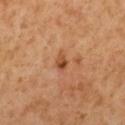Clinical impression:
The lesion was tiled from a total-body skin photograph and was not biopsied.
Clinical summary:
A male patient, in their 60s. The total-body-photography lesion software estimated a shape eccentricity near 0.8. The analysis additionally found a lesion-to-skin contrast of about 6.5 (normalized; higher = more distinct). The recorded lesion diameter is about 3 mm. Imaged with cross-polarized lighting. This image is a 15 mm lesion crop taken from a total-body photograph. The lesion is located on the mid back.